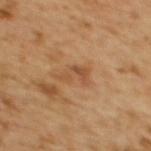Q: Is there a histopathology result?
A: total-body-photography surveillance lesion; no biopsy
Q: What is the lesion's diameter?
A: ≈3.5 mm
Q: Automated lesion metrics?
A: a lesion color around L≈52 a*≈22 b*≈37 in CIELAB, about 8 CIELAB-L* units darker than the surrounding skin, and a lesion-to-skin contrast of about 6 (normalized; higher = more distinct); a border-irregularity index near 5.5/10, a color-variation rating of about 2/10, and a peripheral color-asymmetry measure near 0.5; a classifier nevus-likeness of about 0/100 and lesion-presence confidence of about 100/100
Q: What is the anatomic site?
A: the back
Q: What are the patient's age and sex?
A: female, roughly 55 years of age
Q: What lighting was used for the tile?
A: cross-polarized illumination
Q: What is the imaging modality?
A: ~15 mm crop, total-body skin-cancer survey